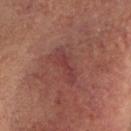Recorded during total-body skin imaging; not selected for excision or biopsy. A female subject, aged around 60. On the head or neck. This is a cross-polarized tile. A 15 mm close-up tile from a total-body photography series done for melanoma screening. Measured at roughly 4.5 mm in maximum diameter.